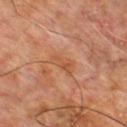Findings:
– workup — total-body-photography surveillance lesion; no biopsy
– patient — male, aged 58–62
– automated lesion analysis — a color-variation rating of about 3/10 and radial color variation of about 1
– location — the upper back
– diameter — about 3.5 mm
– tile lighting — cross-polarized
– image source — ~15 mm crop, total-body skin-cancer survey